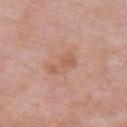The lesion was photographed on a routine skin check and not biopsied; there is no pathology result. Approximately 3.5 mm at its widest. This image is a 15 mm lesion crop taken from a total-body photograph. A male patient aged around 55. This is a white-light tile. From the right upper arm.